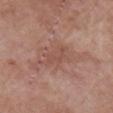Findings:
• notes: no biopsy performed (imaged during a skin exam)
• patient: female, in their 70s
• image: 15 mm crop, total-body photography
• TBP lesion metrics: an outline eccentricity of about 0.7 (0 = round, 1 = elongated); a lesion color around L≈51 a*≈21 b*≈26 in CIELAB, about 6 CIELAB-L* units darker than the surrounding skin, and a normalized lesion–skin contrast near 4.5; a border-irregularity index near 5/10, a within-lesion color-variation index near 2.5/10, and peripheral color asymmetry of about 1
• body site: the arm
• lesion diameter: ≈4.5 mm